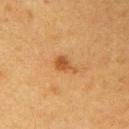workup=catalogued during a skin exam; not biopsied
image=~15 mm tile from a whole-body skin photo
body site=the right upper arm
subject=female, aged 38–42